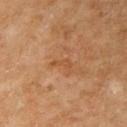Impression:
No biopsy was performed on this lesion — it was imaged during a full skin examination and was not determined to be concerning.
Background:
This image is a 15 mm lesion crop taken from a total-body photograph. Located on the right upper arm. A female subject aged approximately 60. Automated image analysis of the tile measured about 7 CIELAB-L* units darker than the surrounding skin and a lesion-to-skin contrast of about 5.5 (normalized; higher = more distinct). The software also gave a nevus-likeness score of about 0/100. The tile uses cross-polarized illumination. Approximately 2.5 mm at its widest.